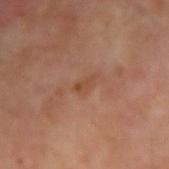Imaged during a routine full-body skin examination; the lesion was not biopsied and no histopathology is available. A male subject aged approximately 70. Captured under cross-polarized illumination. The lesion-visualizer software estimated a border-irregularity rating of about 4/10 and a within-lesion color-variation index near 0/10. The analysis additionally found a detector confidence of about 100 out of 100 that the crop contains a lesion. On the right forearm. A 15 mm close-up tile from a total-body photography series done for melanoma screening.